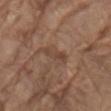follow-up: no biopsy performed (imaged during a skin exam)
anatomic site: the mid back
subject: male, aged 78 to 82
tile lighting: white-light
lesion diameter: ≈3.5 mm
image-analysis metrics: an area of roughly 3 mm², a shape eccentricity near 0.95, and a symmetry-axis asymmetry near 0.35
image: 15 mm crop, total-body photography